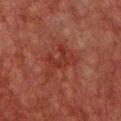workup: imaged on a skin check; not biopsied
image: 15 mm crop, total-body photography
tile lighting: cross-polarized illumination
anatomic site: the chest
size: ≈5 mm
patient: male, in their 60s